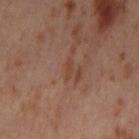Impression:
Part of a total-body skin-imaging series; this lesion was reviewed on a skin check and was not flagged for biopsy.
Context:
The tile uses cross-polarized illumination. Measured at roughly 2.5 mm in maximum diameter. On the left thigh. A female patient aged approximately 55. Cropped from a total-body skin-imaging series; the visible field is about 15 mm.